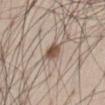Image and clinical context:
A 15 mm close-up extracted from a 3D total-body photography capture. Automated image analysis of the tile measured a footprint of about 5 mm², an outline eccentricity of about 0.65 (0 = round, 1 = elongated), and a shape-asymmetry score of about 0.35 (0 = symmetric). The analysis additionally found a mean CIELAB color near L≈52 a*≈15 b*≈26, about 13 CIELAB-L* units darker than the surrounding skin, and a normalized lesion–skin contrast near 9.5. The software also gave a border-irregularity index near 3.5/10. And it measured a classifier nevus-likeness of about 95/100 and lesion-presence confidence of about 100/100. This is a white-light tile. The lesion is on the abdomen. The lesion's longest dimension is about 3 mm. A male subject aged approximately 60.